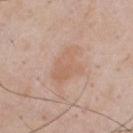  biopsy_status: not biopsied; imaged during a skin examination
  lighting: white-light
  lesion_size:
    long_diameter_mm_approx: 4.5
  patient:
    sex: male
    age_approx: 55
  site: chest
  image:
    source: total-body photography crop
    field_of_view_mm: 15